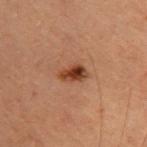  image:
    source: total-body photography crop
    field_of_view_mm: 15
  site: upper back
  patient:
    sex: female
    age_approx: 40
  lesion_size:
    long_diameter_mm_approx: 3.0